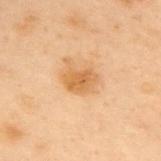The lesion was tiled from a total-body skin photograph and was not biopsied.
Located on the back.
The patient is a male aged approximately 55.
An algorithmic analysis of the crop reported an area of roughly 7 mm², an eccentricity of roughly 0.7, and a symmetry-axis asymmetry near 0.2. The software also gave a mean CIELAB color near L≈52 a*≈18 b*≈37 and a normalized lesion–skin contrast near 7.5.
Imaged with cross-polarized lighting.
A 15 mm close-up extracted from a 3D total-body photography capture.
The recorded lesion diameter is about 3.5 mm.